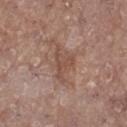Imaged during a routine full-body skin examination; the lesion was not biopsied and no histopathology is available. A roughly 15 mm field-of-view crop from a total-body skin photograph. A female subject, about 75 years old. Measured at roughly 4.5 mm in maximum diameter. The tile uses white-light illumination. The lesion is located on the right lower leg.